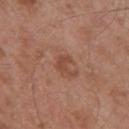Case summary:
- follow-up — imaged on a skin check; not biopsied
- location — the back
- image source — ~15 mm tile from a whole-body skin photo
- size — ≈4.5 mm
- patient — male, in their mid-50s
- image-analysis metrics — an area of roughly 10 mm², an outline eccentricity of about 0.8 (0 = round, 1 = elongated), and a symmetry-axis asymmetry near 0.25; an average lesion color of about L≈50 a*≈21 b*≈29 (CIELAB), roughly 6 lightness units darker than nearby skin, and a normalized lesion–skin contrast near 4.5; a border-irregularity index near 2.5/10 and internal color variation of about 4 on a 0–10 scale; an automated nevus-likeness rating near 0 out of 100 and lesion-presence confidence of about 100/100